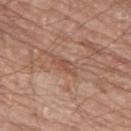No biopsy was performed on this lesion — it was imaged during a full skin examination and was not determined to be concerning.
The recorded lesion diameter is about 3.5 mm.
An algorithmic analysis of the crop reported internal color variation of about 2 on a 0–10 scale and radial color variation of about 0.5.
A male subject, approximately 80 years of age.
This is a white-light tile.
The lesion is located on the right leg.
A lesion tile, about 15 mm wide, cut from a 3D total-body photograph.